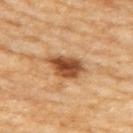The lesion was tiled from a total-body skin photograph and was not biopsied.
From the arm.
The lesion's longest dimension is about 4.5 mm.
Imaged with cross-polarized lighting.
A female patient, aged around 60.
An algorithmic analysis of the crop reported a lesion area of about 9.5 mm² and two-axis asymmetry of about 0.25. The analysis additionally found a mean CIELAB color near L≈50 a*≈24 b*≈38, a lesion–skin lightness drop of about 17, and a normalized lesion–skin contrast near 11. It also reported border irregularity of about 2.5 on a 0–10 scale, internal color variation of about 5 on a 0–10 scale, and a peripheral color-asymmetry measure near 1.5.
A lesion tile, about 15 mm wide, cut from a 3D total-body photograph.